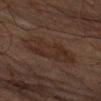{"biopsy_status": "not biopsied; imaged during a skin examination", "image": {"source": "total-body photography crop", "field_of_view_mm": 15}, "automated_metrics": {"border_irregularity_0_10": 3.0, "color_variation_0_10": 2.5, "peripheral_color_asymmetry": 1.0, "nevus_likeness_0_100": 0}, "patient": {"sex": "male", "age_approx": 70}, "site": "left upper arm", "lighting": "cross-polarized"}